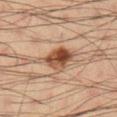Imaged during a routine full-body skin examination; the lesion was not biopsied and no histopathology is available. This is a cross-polarized tile. A male patient, about 35 years old. Located on the right lower leg. Longest diameter approximately 5.5 mm. This image is a 15 mm lesion crop taken from a total-body photograph.